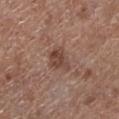Assessment:
No biopsy was performed on this lesion — it was imaged during a full skin examination and was not determined to be concerning.
Image and clinical context:
This image is a 15 mm lesion crop taken from a total-body photograph. Located on the leg. Imaged with white-light lighting. Measured at roughly 3.5 mm in maximum diameter. The patient is a male aged 68–72.